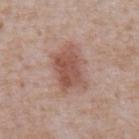biopsy_status: not biopsied; imaged during a skin examination
patient:
  sex: male
  age_approx: 65
image:
  source: total-body photography crop
  field_of_view_mm: 15
lighting: white-light
site: abdomen